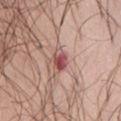Impression: The lesion was tiled from a total-body skin photograph and was not biopsied. Image and clinical context: An algorithmic analysis of the crop reported a border-irregularity rating of about 1.5/10, a color-variation rating of about 6.5/10, and radial color variation of about 2. The analysis additionally found an automated nevus-likeness rating near 0 out of 100 and a detector confidence of about 100 out of 100 that the crop contains a lesion. A 15 mm close-up extracted from a 3D total-body photography capture. The tile uses white-light illumination. A female subject, aged around 55. Measured at roughly 2.5 mm in maximum diameter. Located on the abdomen.